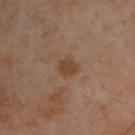Assessment:
This lesion was catalogued during total-body skin photography and was not selected for biopsy.
Context:
Located on the back. Approximately 2.5 mm at its widest. A 15 mm close-up tile from a total-body photography series done for melanoma screening. A male subject in their mid- to late 50s. Imaged with cross-polarized lighting.